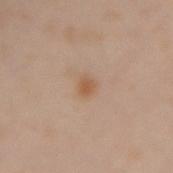Recorded during total-body skin imaging; not selected for excision or biopsy. This image is a 15 mm lesion crop taken from a total-body photograph. A female subject, aged approximately 40. Located on the right forearm. Imaged with cross-polarized lighting.